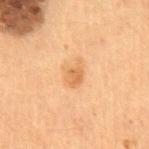Assessment: The lesion was tiled from a total-body skin photograph and was not biopsied.